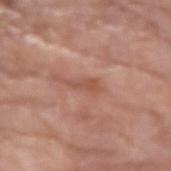{
  "biopsy_status": "not biopsied; imaged during a skin examination",
  "image": {
    "source": "total-body photography crop",
    "field_of_view_mm": 15
  },
  "lesion_size": {
    "long_diameter_mm_approx": 3.5
  },
  "patient": {
    "sex": "female",
    "age_approx": 80
  },
  "site": "right forearm"
}